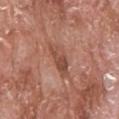Q: Was this lesion biopsied?
A: no biopsy performed (imaged during a skin exam)
Q: Who is the patient?
A: male, aged around 65
Q: How was the tile lit?
A: white-light illumination
Q: Automated lesion metrics?
A: a footprint of about 7.5 mm², an eccentricity of roughly 0.9, and two-axis asymmetry of about 0.3
Q: How was this image acquired?
A: ~15 mm crop, total-body skin-cancer survey
Q: Lesion location?
A: the head or neck